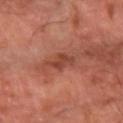workup: catalogued during a skin exam; not biopsied | lesion diameter: ≈3.5 mm | site: the arm | illumination: cross-polarized illumination | patient: about 65 years old | imaging modality: total-body-photography crop, ~15 mm field of view.